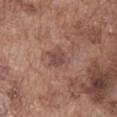Impression: No biopsy was performed on this lesion — it was imaged during a full skin examination and was not determined to be concerning. Acquisition and patient details: The lesion is located on the abdomen. This is a white-light tile. A 15 mm close-up extracted from a 3D total-body photography capture. The total-body-photography lesion software estimated a lesion area of about 5 mm² and an eccentricity of roughly 0.45. The analysis additionally found a border-irregularity rating of about 2/10, a within-lesion color-variation index near 2.5/10, and peripheral color asymmetry of about 1. A male patient, in their mid-70s.